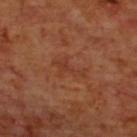This lesion was catalogued during total-body skin photography and was not selected for biopsy. A close-up tile cropped from a whole-body skin photograph, about 15 mm across. The tile uses cross-polarized illumination. A male subject aged 68–72. The total-body-photography lesion software estimated a mean CIELAB color near L≈36 a*≈24 b*≈30, about 5 CIELAB-L* units darker than the surrounding skin, and a normalized border contrast of about 4.5. The analysis additionally found border irregularity of about 7 on a 0–10 scale, a color-variation rating of about 1/10, and radial color variation of about 0. It also reported an automated nevus-likeness rating near 0 out of 100 and lesion-presence confidence of about 100/100. The lesion is located on the upper back. Longest diameter approximately 4 mm.